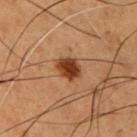Assessment:
No biopsy was performed on this lesion — it was imaged during a full skin examination and was not determined to be concerning.
Clinical summary:
A male subject, about 50 years old. The tile uses cross-polarized illumination. This image is a 15 mm lesion crop taken from a total-body photograph. Approximately 3.5 mm at its widest. Located on the chest.